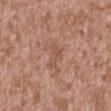This lesion was catalogued during total-body skin photography and was not selected for biopsy. On the chest. Cropped from a total-body skin-imaging series; the visible field is about 15 mm. The recorded lesion diameter is about 3.5 mm. The subject is a male aged 43–47. Captured under white-light illumination.